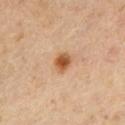This lesion was catalogued during total-body skin photography and was not selected for biopsy. The total-body-photography lesion software estimated an outline eccentricity of about 0.55 (0 = round, 1 = elongated) and a symmetry-axis asymmetry near 0.25. The analysis additionally found about 13 CIELAB-L* units darker than the surrounding skin and a normalized border contrast of about 9.5. The software also gave a within-lesion color-variation index near 5.5/10. Approximately 2.5 mm at its widest. The lesion is located on the left upper arm. A male subject, roughly 55 years of age. Captured under cross-polarized illumination. A close-up tile cropped from a whole-body skin photograph, about 15 mm across.